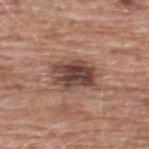imaging modality: total-body-photography crop, ~15 mm field of view
patient: male, approximately 60 years of age
automated lesion analysis: a lesion area of about 14 mm² and an outline eccentricity of about 0.75 (0 = round, 1 = elongated); a border-irregularity index near 2.5/10 and internal color variation of about 7 on a 0–10 scale
location: the upper back